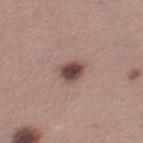Imaged during a routine full-body skin examination; the lesion was not biopsied and no histopathology is available. The patient is a female approximately 30 years of age. Cropped from a whole-body photographic skin survey; the tile spans about 15 mm. Located on the left thigh.